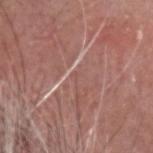{"diagnosis": {"histopathology": "seborrheic keratosis", "malignancy": "benign", "taxonomic_path": ["Benign", "Benign epidermal proliferations", "Seborrheic keratosis"]}}